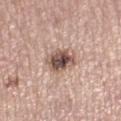Clinical impression:
Imaged during a routine full-body skin examination; the lesion was not biopsied and no histopathology is available.
Image and clinical context:
Imaged with white-light lighting. A 15 mm crop from a total-body photograph taken for skin-cancer surveillance. Measured at roughly 3.5 mm in maximum diameter. On the left lower leg. The total-body-photography lesion software estimated an average lesion color of about L≈52 a*≈18 b*≈23 (CIELAB) and roughly 17 lightness units darker than nearby skin. The software also gave border irregularity of about 2 on a 0–10 scale, a color-variation rating of about 8.5/10, and radial color variation of about 2.5. It also reported a classifier nevus-likeness of about 90/100. A female subject about 65 years old.